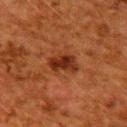follow-up: imaged on a skin check; not biopsied
patient: female, aged 48–52
body site: the upper back
image-analysis metrics: an area of roughly 6.5 mm², an outline eccentricity of about 0.75 (0 = round, 1 = elongated), and a symmetry-axis asymmetry near 0.3; a mean CIELAB color near L≈26 a*≈24 b*≈30, about 10 CIELAB-L* units darker than the surrounding skin, and a normalized border contrast of about 10
lighting: cross-polarized illumination
imaging modality: 15 mm crop, total-body photography